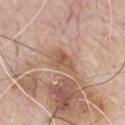notes: imaged on a skin check; not biopsied | patient: male, roughly 80 years of age | diameter: about 3.5 mm | body site: the chest | tile lighting: white-light | image source: ~15 mm tile from a whole-body skin photo.